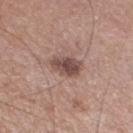<lesion>
<biopsy_status>not biopsied; imaged during a skin examination</biopsy_status>
<site>left lower leg</site>
<lesion_size>
  <long_diameter_mm_approx>3.5</long_diameter_mm_approx>
</lesion_size>
<patient>
  <sex>male</sex>
  <age_approx>50</age_approx>
</patient>
<image>
  <source>total-body photography crop</source>
  <field_of_view_mm>15</field_of_view_mm>
</image>
</lesion>